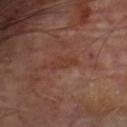workup — total-body-photography surveillance lesion; no biopsy
acquisition — total-body-photography crop, ~15 mm field of view
site — the chest
patient — male, in their mid- to late 60s